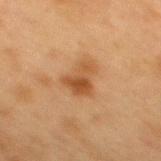{
  "image": {
    "source": "total-body photography crop",
    "field_of_view_mm": 15
  },
  "site": "mid back",
  "lesion_size": {
    "long_diameter_mm_approx": 4.0
  },
  "patient": {
    "sex": "female",
    "age_approx": 40
  },
  "lighting": "cross-polarized"
}